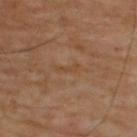image source=~15 mm tile from a whole-body skin photo
body site=the upper back
tile lighting=cross-polarized
lesion diameter=~3 mm (longest diameter)
image-analysis metrics=internal color variation of about 0 on a 0–10 scale and radial color variation of about 0; a nevus-likeness score of about 0/100
patient=male, in their mid- to late 60s